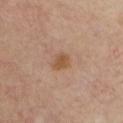Captured during whole-body skin photography for melanoma surveillance; the lesion was not biopsied.
The patient is a male aged 53 to 57.
The lesion is on the chest.
Captured under cross-polarized illumination.
A roughly 15 mm field-of-view crop from a total-body skin photograph.